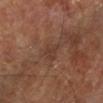This lesion was catalogued during total-body skin photography and was not selected for biopsy. A patient aged around 65. Located on the left lower leg. This image is a 15 mm lesion crop taken from a total-body photograph. The total-body-photography lesion software estimated an automated nevus-likeness rating near 0 out of 100 and lesion-presence confidence of about 90/100.